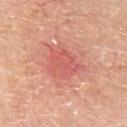{"biopsy_status": "not biopsied; imaged during a skin examination", "image": {"source": "total-body photography crop", "field_of_view_mm": 15}, "lighting": "white-light", "site": "chest", "lesion_size": {"long_diameter_mm_approx": 4.5}, "patient": {"sex": "male", "age_approx": 80}}